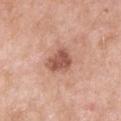Clinical impression:
The lesion was photographed on a routine skin check and not biopsied; there is no pathology result.
Background:
Cropped from a whole-body photographic skin survey; the tile spans about 15 mm. Measured at roughly 3.5 mm in maximum diameter. The lesion is located on the left upper arm. Captured under white-light illumination. A male patient in their mid-50s. Automated image analysis of the tile measured a border-irregularity index near 2.5/10 and radial color variation of about 1.